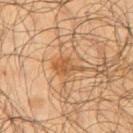Imaged during a routine full-body skin examination; the lesion was not biopsied and no histopathology is available.
The patient is a male approximately 65 years of age.
Located on the right upper arm.
A 15 mm crop from a total-body photograph taken for skin-cancer surveillance.